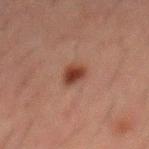Clinical impression:
Recorded during total-body skin imaging; not selected for excision or biopsy.
Context:
A close-up tile cropped from a whole-body skin photograph, about 15 mm across. This is a cross-polarized tile. An algorithmic analysis of the crop reported a footprint of about 5 mm², a shape eccentricity near 0.55, and a shape-asymmetry score of about 0.25 (0 = symmetric). It also reported a border-irregularity index near 2.5/10, internal color variation of about 3 on a 0–10 scale, and radial color variation of about 1. And it measured a classifier nevus-likeness of about 100/100 and lesion-presence confidence of about 100/100. The subject is a male aged around 50. The lesion is on the mid back. About 3 mm across.